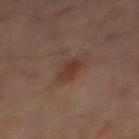workup = no biopsy performed (imaged during a skin exam); acquisition = ~15 mm tile from a whole-body skin photo; subject = male, aged 58 to 62; illumination = cross-polarized illumination; anatomic site = the mid back.